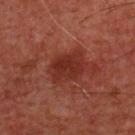An algorithmic analysis of the crop reported a classifier nevus-likeness of about 0/100 and a lesion-detection confidence of about 100/100. Captured under cross-polarized illumination. The lesion's longest dimension is about 4.5 mm. Cropped from a whole-body photographic skin survey; the tile spans about 15 mm. On the chest. A male subject aged around 60.